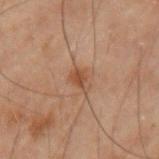Part of a total-body skin-imaging series; this lesion was reviewed on a skin check and was not flagged for biopsy. A male patient aged 68 to 72. The tile uses cross-polarized illumination. A 15 mm close-up extracted from a 3D total-body photography capture. On the left upper arm.